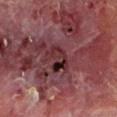A male subject, roughly 70 years of age.
From the left lower leg.
This is a cross-polarized tile.
The lesion's longest dimension is about 3 mm.
A roughly 15 mm field-of-view crop from a total-body skin photograph.